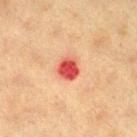Imaged during a routine full-body skin examination; the lesion was not biopsied and no histopathology is available. The lesion is on the right thigh. Captured under cross-polarized illumination. The subject is a female aged 38 to 42. Cropped from a total-body skin-imaging series; the visible field is about 15 mm.